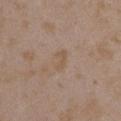A female subject in their mid- to late 30s. The lesion is on the right upper arm. The recorded lesion diameter is about 2.5 mm. The tile uses white-light illumination. A 15 mm close-up extracted from a 3D total-body photography capture.